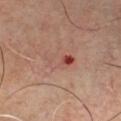Recorded during total-body skin imaging; not selected for excision or biopsy. A roughly 15 mm field-of-view crop from a total-body skin photograph. About 4 mm across. The subject is aged approximately 65. On the chest.